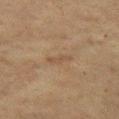Assessment: Imaged during a routine full-body skin examination; the lesion was not biopsied and no histopathology is available. Image and clinical context: Imaged with cross-polarized lighting. From the left thigh. Cropped from a total-body skin-imaging series; the visible field is about 15 mm. A female subject aged approximately 40.